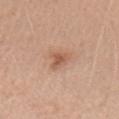Part of a total-body skin-imaging series; this lesion was reviewed on a skin check and was not flagged for biopsy.
A 15 mm crop from a total-body photograph taken for skin-cancer surveillance.
Located on the left upper arm.
A male subject aged 48 to 52.
The tile uses white-light illumination.
The lesion's longest dimension is about 3 mm.